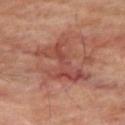Recorded during total-body skin imaging; not selected for excision or biopsy.
A male patient, aged approximately 70.
Located on the right thigh.
Automated image analysis of the tile measured a mean CIELAB color near L≈47 a*≈26 b*≈27. And it measured border irregularity of about 8.5 on a 0–10 scale, a color-variation rating of about 5.5/10, and peripheral color asymmetry of about 2. The analysis additionally found a nevus-likeness score of about 0/100 and a lesion-detection confidence of about 95/100.
Imaged with cross-polarized lighting.
A lesion tile, about 15 mm wide, cut from a 3D total-body photograph.